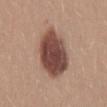Case summary:
- notes: catalogued during a skin exam; not biopsied
- diameter: ~7 mm (longest diameter)
- patient: female, aged 23–27
- site: the back
- acquisition: ~15 mm crop, total-body skin-cancer survey
- tile lighting: white-light